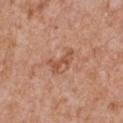<lesion>
  <biopsy_status>not biopsied; imaged during a skin examination</biopsy_status>
  <patient>
    <sex>male</sex>
    <age_approx>65</age_approx>
  </patient>
  <lesion_size>
    <long_diameter_mm_approx>3.5</long_diameter_mm_approx>
  </lesion_size>
  <image>
    <source>total-body photography crop</source>
    <field_of_view_mm>15</field_of_view_mm>
  </image>
  <site>chest</site>
  <lighting>white-light</lighting>
  <automated_metrics>
    <cielab_L>53</cielab_L>
    <cielab_a>25</cielab_a>
    <cielab_b>33</cielab_b>
    <vs_skin_darker_L>9.0</vs_skin_darker_L>
    <vs_skin_contrast_norm>6.5</vs_skin_contrast_norm>
    <nevus_likeness_0_100>0</nevus_likeness_0_100>
    <lesion_detection_confidence_0_100>100</lesion_detection_confidence_0_100>
  </automated_metrics>
</lesion>